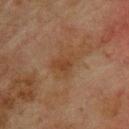workup = no biopsy performed (imaged during a skin exam)
size = about 4 mm
anatomic site = the back
lighting = cross-polarized
patient = male, roughly 75 years of age
automated metrics = a lesion area of about 7.5 mm², a shape eccentricity near 0.75, and two-axis asymmetry of about 0.35; a border-irregularity index near 4/10 and internal color variation of about 3 on a 0–10 scale; a nevus-likeness score of about 0/100 and lesion-presence confidence of about 100/100
acquisition = ~15 mm tile from a whole-body skin photo